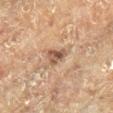Findings:
- follow-up: imaged on a skin check; not biopsied
- image-analysis metrics: a lesion color around L≈42 a*≈15 b*≈25 in CIELAB, roughly 10 lightness units darker than nearby skin, and a normalized border contrast of about 8; a border-irregularity rating of about 3.5/10 and a within-lesion color-variation index near 5.5/10; a lesion-detection confidence of about 100/100
- tile lighting: cross-polarized
- patient: male, about 75 years old
- diameter: about 3 mm
- body site: the right lower leg
- acquisition: total-body-photography crop, ~15 mm field of view